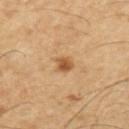notes=catalogued during a skin exam; not biopsied
body site=the back
automated lesion analysis=an average lesion color of about L≈52 a*≈21 b*≈38 (CIELAB) and a normalized border contrast of about 8.5; a color-variation rating of about 2.5/10 and peripheral color asymmetry of about 1
illumination=cross-polarized illumination
size=≈2 mm
subject=male, aged 58 to 62
acquisition=~15 mm tile from a whole-body skin photo